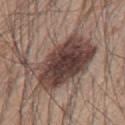<record>
  <biopsy_status>not biopsied; imaged during a skin examination</biopsy_status>
  <site>mid back</site>
  <automated_metrics>
    <cielab_L>41</cielab_L>
    <cielab_a>16</cielab_a>
    <cielab_b>20</cielab_b>
    <vs_skin_darker_L>15.0</vs_skin_darker_L>
    <vs_skin_contrast_norm>12.0</vs_skin_contrast_norm>
  </automated_metrics>
  <lesion_size>
    <long_diameter_mm_approx>8.5</long_diameter_mm_approx>
  </lesion_size>
  <image>
    <source>total-body photography crop</source>
    <field_of_view_mm>15</field_of_view_mm>
  </image>
  <patient>
    <sex>male</sex>
    <age_approx>60</age_approx>
  </patient>
</record>